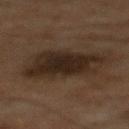biopsy status = no biopsy performed (imaged during a skin exam) | anatomic site = the front of the torso | subject = male, roughly 60 years of age | tile lighting = cross-polarized | acquisition = 15 mm crop, total-body photography.